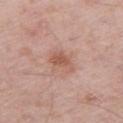Impression: Recorded during total-body skin imaging; not selected for excision or biopsy. Background: The lesion-visualizer software estimated a mean CIELAB color near L≈57 a*≈22 b*≈28, roughly 9 lightness units darker than nearby skin, and a lesion-to-skin contrast of about 6.5 (normalized; higher = more distinct). And it measured an automated nevus-likeness rating near 50 out of 100 and a detector confidence of about 100 out of 100 that the crop contains a lesion. This image is a 15 mm lesion crop taken from a total-body photograph. A male patient in their 60s. The lesion is located on the left thigh.